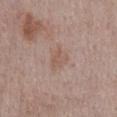location: the chest
size: ≈4 mm
image-analysis metrics: a lesion area of about 7.5 mm² and a shape-asymmetry score of about 0.3 (0 = symmetric); roughly 6 lightness units darker than nearby skin and a normalized border contrast of about 5; a nevus-likeness score of about 0/100 and a detector confidence of about 100 out of 100 that the crop contains a lesion
acquisition: ~15 mm crop, total-body skin-cancer survey
patient: male, in their mid- to late 50s